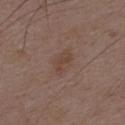Assessment: The lesion was tiled from a total-body skin photograph and was not biopsied. Acquisition and patient details: A male subject aged approximately 50. From the back. An algorithmic analysis of the crop reported an area of roughly 7.5 mm², an eccentricity of roughly 0.75, and a symmetry-axis asymmetry near 0.25. The software also gave an average lesion color of about L≈44 a*≈16 b*≈24 (CIELAB) and a lesion-to-skin contrast of about 4.5 (normalized; higher = more distinct). The software also gave a nevus-likeness score of about 0/100 and lesion-presence confidence of about 100/100. A 15 mm close-up tile from a total-body photography series done for melanoma screening.